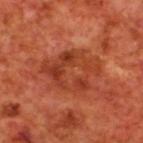  patient:
    sex: male
    age_approx: 70
  site: upper back
  image:
    source: total-body photography crop
    field_of_view_mm: 15
  lighting: cross-polarized
  automated_metrics:
    area_mm2_approx: 22.0
    eccentricity: 0.65
    cielab_L: 40
    cielab_a: 31
    cielab_b: 36
    vs_skin_contrast_norm: 6.0